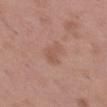Captured during whole-body skin photography for melanoma surveillance; the lesion was not biopsied. Captured under white-light illumination. The patient is a male aged approximately 50. Cropped from a whole-body photographic skin survey; the tile spans about 15 mm. About 3 mm across. On the left thigh.